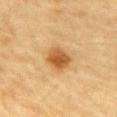biopsy status = no biopsy performed (imaged during a skin exam)
site = the abdomen
diameter = about 3.5 mm
lighting = cross-polarized illumination
TBP lesion metrics = a normalized lesion–skin contrast near 8.5; an automated nevus-likeness rating near 95 out of 100 and a lesion-detection confidence of about 100/100
image source = total-body-photography crop, ~15 mm field of view
subject = male, about 85 years old or older